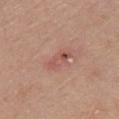Q: Was a biopsy performed?
A: total-body-photography surveillance lesion; no biopsy
Q: What are the patient's age and sex?
A: male, about 60 years old
Q: What is the anatomic site?
A: the chest
Q: Illumination type?
A: white-light
Q: What did automated image analysis measure?
A: border irregularity of about 7 on a 0–10 scale, internal color variation of about 0 on a 0–10 scale, and peripheral color asymmetry of about 0; a nevus-likeness score of about 0/100
Q: What kind of image is this?
A: ~15 mm crop, total-body skin-cancer survey